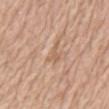Impression: Recorded during total-body skin imaging; not selected for excision or biopsy. Background: Cropped from a total-body skin-imaging series; the visible field is about 15 mm. A male subject, aged around 70. The lesion is located on the mid back. The total-body-photography lesion software estimated a lesion area of about 3 mm² and an eccentricity of roughly 0.75. And it measured a border-irregularity index near 6.5/10, a color-variation rating of about 0/10, and peripheral color asymmetry of about 0. This is a white-light tile.